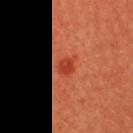Clinical impression: Imaged during a routine full-body skin examination; the lesion was not biopsied and no histopathology is available. Background: About 2.5 mm across. The tile uses cross-polarized illumination. The lesion is on the head or neck. This image is a 15 mm lesion crop taken from a total-body photograph. A male patient, aged 38–42.